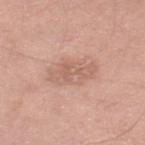follow-up: catalogued during a skin exam; not biopsied
body site: the right thigh
subject: male, in their 50s
imaging modality: ~15 mm tile from a whole-body skin photo